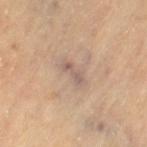{"biopsy_status": "not biopsied; imaged during a skin examination", "site": "leg", "lesion_size": {"long_diameter_mm_approx": 3.5}, "lighting": "cross-polarized", "patient": {"sex": "female", "age_approx": 70}, "automated_metrics": {"area_mm2_approx": 4.0, "eccentricity": 0.9, "shape_asymmetry": 0.35, "cielab_L": 60, "cielab_a": 16, "cielab_b": 25, "vs_skin_darker_L": 8.0, "vs_skin_contrast_norm": 6.5, "color_variation_0_10": 1.5, "peripheral_color_asymmetry": 0.5, "nevus_likeness_0_100": 0}, "image": {"source": "total-body photography crop", "field_of_view_mm": 15}}